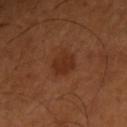Captured during whole-body skin photography for melanoma surveillance; the lesion was not biopsied. A male patient about 50 years old. The lesion is on the right upper arm. A roughly 15 mm field-of-view crop from a total-body skin photograph.